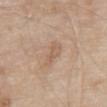About 3.5 mm across. The total-body-photography lesion software estimated an outline eccentricity of about 0.9 (0 = round, 1 = elongated) and two-axis asymmetry of about 0.4. The software also gave a mean CIELAB color near L≈59 a*≈17 b*≈30 and about 7 CIELAB-L* units darker than the surrounding skin. It also reported a within-lesion color-variation index near 2.5/10 and a peripheral color-asymmetry measure near 1. The analysis additionally found a nevus-likeness score of about 0/100. Imaged with white-light lighting. A roughly 15 mm field-of-view crop from a total-body skin photograph. A male subject, about 80 years old. From the abdomen.